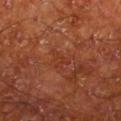The lesion was tiled from a total-body skin photograph and was not biopsied.